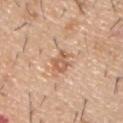No biopsy was performed on this lesion — it was imaged during a full skin examination and was not determined to be concerning.
A male subject, in their 60s.
Located on the upper back.
About 2.5 mm across.
Automated image analysis of the tile measured a mean CIELAB color near L≈60 a*≈22 b*≈33, about 10 CIELAB-L* units darker than the surrounding skin, and a normalized border contrast of about 6.5. The analysis additionally found a color-variation rating of about 3/10 and a peripheral color-asymmetry measure near 1.
Imaged with white-light lighting.
A 15 mm close-up tile from a total-body photography series done for melanoma screening.